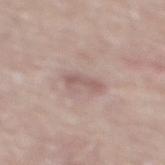diameter: ~3.5 mm (longest diameter) | acquisition: 15 mm crop, total-body photography | tile lighting: white-light illumination | subject: male, aged approximately 80 | location: the mid back.